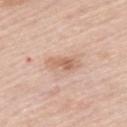• biopsy status: no biopsy performed (imaged during a skin exam)
• imaging modality: total-body-photography crop, ~15 mm field of view
• subject: male, about 60 years old
• body site: the back
• illumination: white-light illumination